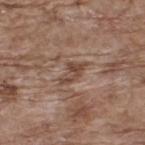imaging modality: ~15 mm crop, total-body skin-cancer survey; anatomic site: the upper back; patient: male, approximately 70 years of age.